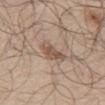| feature | finding |
|---|---|
| notes | imaged on a skin check; not biopsied |
| illumination | white-light |
| automated metrics | a footprint of about 5.5 mm² and two-axis asymmetry of about 0.4; an automated nevus-likeness rating near 25 out of 100 and a detector confidence of about 90 out of 100 that the crop contains a lesion |
| lesion diameter | ≈4 mm |
| image source | 15 mm crop, total-body photography |
| anatomic site | the abdomen |
| subject | male, approximately 75 years of age |